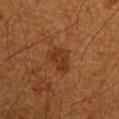| field | value |
|---|---|
| notes | total-body-photography surveillance lesion; no biopsy |
| patient | male, aged 58–62 |
| location | the left upper arm |
| automated lesion analysis | a border-irregularity index near 3.5/10, a color-variation rating of about 2.5/10, and a peripheral color-asymmetry measure near 0.5 |
| illumination | cross-polarized |
| acquisition | ~15 mm tile from a whole-body skin photo |